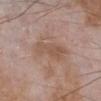notes = imaged on a skin check; not biopsied
imaging modality = ~15 mm crop, total-body skin-cancer survey
subject = male, roughly 65 years of age
illumination = white-light
anatomic site = the leg